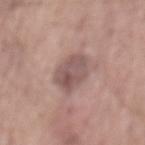{"biopsy_status": "not biopsied; imaged during a skin examination", "site": "mid back", "patient": {"sex": "male", "age_approx": 55}, "lesion_size": {"long_diameter_mm_approx": 5.0}, "automated_metrics": {"area_mm2_approx": 11.0, "eccentricity": 0.75, "shape_asymmetry": 0.2, "cielab_L": 53, "cielab_a": 18, "cielab_b": 20, "vs_skin_darker_L": 11.0, "border_irregularity_0_10": 2.0, "color_variation_0_10": 4.5, "peripheral_color_asymmetry": 2.0}, "image": {"source": "total-body photography crop", "field_of_view_mm": 15}, "lighting": "white-light"}